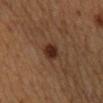Notes:
* notes · catalogued during a skin exam; not biopsied
* site · the mid back
* image · total-body-photography crop, ~15 mm field of view
* patient · female, approximately 55 years of age
* image-analysis metrics · a lesion–skin lightness drop of about 10 and a normalized lesion–skin contrast near 11; a border-irregularity rating of about 2/10 and radial color variation of about 0.5